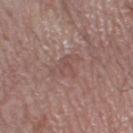{"biopsy_status": "not biopsied; imaged during a skin examination", "lesion_size": {"long_diameter_mm_approx": 3.5}, "image": {"source": "total-body photography crop", "field_of_view_mm": 15}, "site": "left lower leg", "patient": {"sex": "male", "age_approx": 60}}